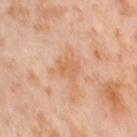Q: Was a biopsy performed?
A: no biopsy performed (imaged during a skin exam)
Q: What are the patient's age and sex?
A: female, roughly 55 years of age
Q: How was the tile lit?
A: cross-polarized
Q: Lesion size?
A: ≈2.5 mm
Q: Lesion location?
A: the leg
Q: What did automated image analysis measure?
A: a mean CIELAB color near L≈64 a*≈23 b*≈37 and a normalized border contrast of about 5.5; an automated nevus-likeness rating near 0 out of 100 and a detector confidence of about 100 out of 100 that the crop contains a lesion
Q: What is the imaging modality?
A: ~15 mm crop, total-body skin-cancer survey